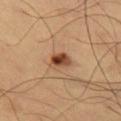follow-up = no biopsy performed (imaged during a skin exam) | TBP lesion metrics = an average lesion color of about L≈46 a*≈22 b*≈33 (CIELAB), about 14 CIELAB-L* units darker than the surrounding skin, and a normalized lesion–skin contrast near 10.5; an automated nevus-likeness rating near 95 out of 100 and lesion-presence confidence of about 100/100 | subject = male, about 60 years old | illumination = cross-polarized illumination | acquisition = 15 mm crop, total-body photography | anatomic site = the left thigh | lesion size = ≈3 mm.